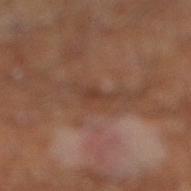{"biopsy_status": "not biopsied; imaged during a skin examination", "lesion_size": {"long_diameter_mm_approx": 2.5}, "image": {"source": "total-body photography crop", "field_of_view_mm": 15}, "patient": {"sex": "male", "age_approx": 60}, "lighting": "cross-polarized", "site": "right lower leg", "automated_metrics": {"cielab_L": 34, "cielab_a": 18, "cielab_b": 25, "vs_skin_darker_L": 6.0, "vs_skin_contrast_norm": 6.0, "color_variation_0_10": 0.5, "peripheral_color_asymmetry": 0.0, "nevus_likeness_0_100": 0}}